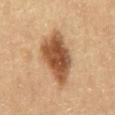  biopsy_status: not biopsied; imaged during a skin examination
  patient:
    sex: male
    age_approx: 60
  lighting: cross-polarized
  image:
    source: total-body photography crop
    field_of_view_mm: 15
  lesion_size:
    long_diameter_mm_approx: 6.5